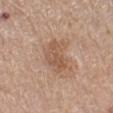Notes:
• biopsy status · total-body-photography surveillance lesion; no biopsy
• subject · female, in their 60s
• diameter · ≈4 mm
• image-analysis metrics · a lesion area of about 10 mm² and a shape-asymmetry score of about 0.3 (0 = symmetric); a nevus-likeness score of about 0/100 and a lesion-detection confidence of about 100/100
• body site · the left lower leg
• illumination · white-light illumination
• image · 15 mm crop, total-body photography